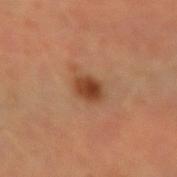biopsy_status: not biopsied; imaged during a skin examination
automated_metrics:
  area_mm2_approx: 5.0
  eccentricity: 0.65
  shape_asymmetry: 0.2
  nevus_likeness_0_100: 95
patient:
  sex: male
  age_approx: 40
site: right forearm
lighting: cross-polarized
lesion_size:
  long_diameter_mm_approx: 3.0
image:
  source: total-body photography crop
  field_of_view_mm: 15